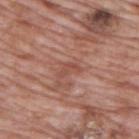Clinical impression: The lesion was photographed on a routine skin check and not biopsied; there is no pathology result. Acquisition and patient details: The lesion's longest dimension is about 2.5 mm. A male patient, about 70 years old. The tile uses white-light illumination. A region of skin cropped from a whole-body photographic capture, roughly 15 mm wide. The lesion-visualizer software estimated a mean CIELAB color near L≈49 a*≈24 b*≈27, a lesion–skin lightness drop of about 7, and a normalized lesion–skin contrast near 5. The software also gave a border-irregularity index near 6.5/10, a within-lesion color-variation index near 0/10, and peripheral color asymmetry of about 0. And it measured a classifier nevus-likeness of about 0/100 and a lesion-detection confidence of about 90/100. The lesion is on the upper back.